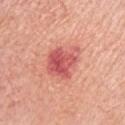The lesion was tiled from a total-body skin photograph and was not biopsied. The patient is a female aged 63–67. Captured under white-light illumination. Measured at roughly 4.5 mm in maximum diameter. Cropped from a whole-body photographic skin survey; the tile spans about 15 mm. On the arm.